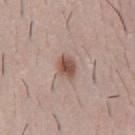Clinical impression: Captured during whole-body skin photography for melanoma surveillance; the lesion was not biopsied. Image and clinical context: The lesion's longest dimension is about 3 mm. A close-up tile cropped from a whole-body skin photograph, about 15 mm across. Automated image analysis of the tile measured an average lesion color of about L≈52 a*≈19 b*≈25 (CIELAB), about 13 CIELAB-L* units darker than the surrounding skin, and a lesion-to-skin contrast of about 9 (normalized; higher = more distinct). It also reported a border-irregularity rating of about 2.5/10, a within-lesion color-variation index near 4/10, and peripheral color asymmetry of about 1.5. The patient is a male aged approximately 40. Located on the chest.